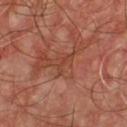Clinical impression: The lesion was photographed on a routine skin check and not biopsied; there is no pathology result. Background: The lesion is located on the chest. Longest diameter approximately 3 mm. A roughly 15 mm field-of-view crop from a total-body skin photograph. A male subject, aged 53 to 57.